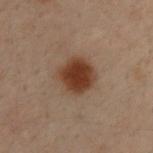Q: Was a biopsy performed?
A: total-body-photography surveillance lesion; no biopsy
Q: What lighting was used for the tile?
A: cross-polarized
Q: How was this image acquired?
A: 15 mm crop, total-body photography
Q: What is the lesion's diameter?
A: ≈4 mm
Q: Automated lesion metrics?
A: a footprint of about 12 mm² and a symmetry-axis asymmetry near 0.15; a lesion color around L≈31 a*≈17 b*≈24 in CIELAB and a normalized border contrast of about 11
Q: Patient demographics?
A: male, about 30 years old
Q: Lesion location?
A: the upper back